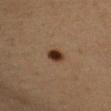Notes:
• notes — catalogued during a skin exam; not biopsied
• lesion diameter — about 2.5 mm
• patient — female, aged 43–47
• acquisition — ~15 mm crop, total-body skin-cancer survey
• location — the left upper arm